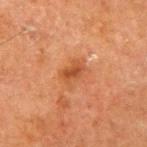Findings:
* biopsy status — catalogued during a skin exam; not biopsied
* site — the right upper arm
* size — ≈3.5 mm
* patient — male, in their mid-70s
* image source — ~15 mm crop, total-body skin-cancer survey